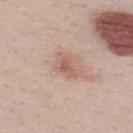Imaged during a routine full-body skin examination; the lesion was not biopsied and no histopathology is available.